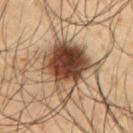The lesion was tiled from a total-body skin photograph and was not biopsied. Longest diameter approximately 7.5 mm. A lesion tile, about 15 mm wide, cut from a 3D total-body photograph. The lesion is on the upper back. The tile uses cross-polarized illumination. A male patient aged 48 to 52.